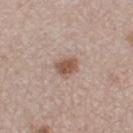This lesion was catalogued during total-body skin photography and was not selected for biopsy. The patient is a male aged 53 to 57. On the left thigh. Cropped from a whole-body photographic skin survey; the tile spans about 15 mm.